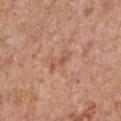Clinical impression:
Part of a total-body skin-imaging series; this lesion was reviewed on a skin check and was not flagged for biopsy.
Context:
Located on the chest. The subject is a male aged approximately 65. A 15 mm close-up tile from a total-body photography series done for melanoma screening.